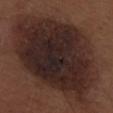Captured during whole-body skin photography for melanoma surveillance; the lesion was not biopsied. A male subject, about 30 years old. Measured at roughly 13.5 mm in maximum diameter. A lesion tile, about 15 mm wide, cut from a 3D total-body photograph. The tile uses white-light illumination. The lesion is located on the back.